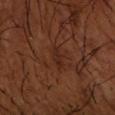{"biopsy_status": "not biopsied; imaged during a skin examination", "image": {"source": "total-body photography crop", "field_of_view_mm": 15}, "site": "left forearm", "patient": {"sex": "male", "age_approx": 65}, "lesion_size": {"long_diameter_mm_approx": 2.5}, "automated_metrics": {"eccentricity": 0.9, "cielab_L": 25, "cielab_a": 21, "cielab_b": 26, "vs_skin_darker_L": 5.0, "nevus_likeness_0_100": 0, "lesion_detection_confidence_0_100": 100}}